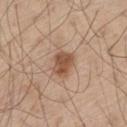Q: Was this lesion biopsied?
A: no biopsy performed (imaged during a skin exam)
Q: What is the lesion's diameter?
A: ≈3 mm
Q: How was this image acquired?
A: total-body-photography crop, ~15 mm field of view
Q: What did automated image analysis measure?
A: an area of roughly 6.5 mm² and a shape-asymmetry score of about 0.25 (0 = symmetric); a border-irregularity rating of about 2/10 and internal color variation of about 2.5 on a 0–10 scale; an automated nevus-likeness rating near 85 out of 100 and a detector confidence of about 100 out of 100 that the crop contains a lesion
Q: Patient demographics?
A: male, aged around 60
Q: Lesion location?
A: the left thigh
Q: How was the tile lit?
A: white-light